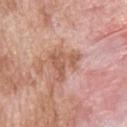Imaged during a routine full-body skin examination; the lesion was not biopsied and no histopathology is available. The lesion's longest dimension is about 4 mm. This is a white-light tile. Located on the upper back. A roughly 15 mm field-of-view crop from a total-body skin photograph. The patient is a male aged approximately 60.